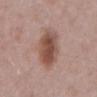Context:
The lesion is located on the abdomen. A 15 mm crop from a total-body photograph taken for skin-cancer surveillance. Captured under white-light illumination. The patient is a male aged 73 to 77.